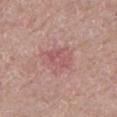notes: imaged on a skin check; not biopsied.